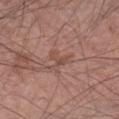Clinical impression:
Recorded during total-body skin imaging; not selected for excision or biopsy.
Acquisition and patient details:
The lesion's longest dimension is about 2.5 mm. Cropped from a total-body skin-imaging series; the visible field is about 15 mm. The lesion is located on the left forearm. The lesion-visualizer software estimated a lesion area of about 3 mm², a shape eccentricity near 0.8, and a shape-asymmetry score of about 0.5 (0 = symmetric). And it measured a peripheral color-asymmetry measure near 0. It also reported a classifier nevus-likeness of about 0/100 and a lesion-detection confidence of about 100/100. A male subject approximately 60 years of age. This is a white-light tile.